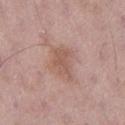Impression: Captured during whole-body skin photography for melanoma surveillance; the lesion was not biopsied.